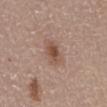Recorded during total-body skin imaging; not selected for excision or biopsy. A lesion tile, about 15 mm wide, cut from a 3D total-body photograph. A female subject aged 63 to 67. The lesion is located on the front of the torso.